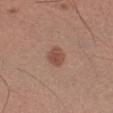biopsy_status: not biopsied; imaged during a skin examination
patient:
  sex: male
  age_approx: 35
site: left forearm
image:
  source: total-body photography crop
  field_of_view_mm: 15
lesion_size:
  long_diameter_mm_approx: 2.5